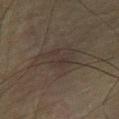{"biopsy_status": "not biopsied; imaged during a skin examination", "site": "chest", "image": {"source": "total-body photography crop", "field_of_view_mm": 15}, "patient": {"sex": "male", "age_approx": 75}, "lighting": "cross-polarized", "lesion_size": {"long_diameter_mm_approx": 5.5}}